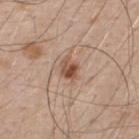Imaged during a routine full-body skin examination; the lesion was not biopsied and no histopathology is available. This is a white-light tile. Located on the back. A male patient, approximately 50 years of age. A close-up tile cropped from a whole-body skin photograph, about 15 mm across.